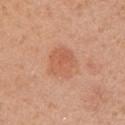<case>
  <biopsy_status>not biopsied; imaged during a skin examination</biopsy_status>
  <site>upper back</site>
  <patient>
    <sex>female</sex>
    <age_approx>25</age_approx>
  </patient>
  <image>
    <source>total-body photography crop</source>
    <field_of_view_mm>15</field_of_view_mm>
  </image>
</case>